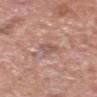Part of a total-body skin-imaging series; this lesion was reviewed on a skin check and was not flagged for biopsy.
About 3.5 mm across.
Automated image analysis of the tile measured a border-irregularity rating of about 4.5/10 and peripheral color asymmetry of about 0.
A 15 mm close-up extracted from a 3D total-body photography capture.
Located on the head or neck.
A male patient aged 58 to 62.